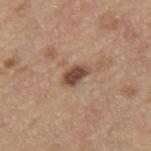biopsy status: no biopsy performed (imaged during a skin exam) | location: the mid back | subject: male, aged around 70 | acquisition: ~15 mm tile from a whole-body skin photo.